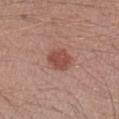Findings:
• biopsy status: no biopsy performed (imaged during a skin exam)
• tile lighting: white-light
• location: the left forearm
• patient: male, aged 38 to 42
• image source: ~15 mm tile from a whole-body skin photo